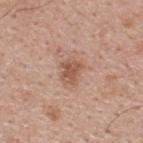<tbp_lesion>
  <biopsy_status>not biopsied; imaged during a skin examination</biopsy_status>
  <lighting>white-light</lighting>
  <image>
    <source>total-body photography crop</source>
    <field_of_view_mm>15</field_of_view_mm>
  </image>
  <automated_metrics>
    <nevus_likeness_0_100>75</nevus_likeness_0_100>
    <lesion_detection_confidence_0_100>100</lesion_detection_confidence_0_100>
  </automated_metrics>
  <patient>
    <sex>male</sex>
    <age_approx>40</age_approx>
  </patient>
  <site>upper back</site>
  <lesion_size>
    <long_diameter_mm_approx>3.0</long_diameter_mm_approx>
  </lesion_size>
</tbp_lesion>